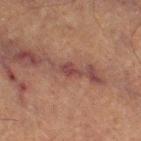follow-up: imaged on a skin check; not biopsied | automated metrics: a lesion area of about 3 mm² and an eccentricity of roughly 0.85; a detector confidence of about 85 out of 100 that the crop contains a lesion | acquisition: ~15 mm crop, total-body skin-cancer survey | subject: female, approximately 60 years of age | body site: the right thigh | tile lighting: cross-polarized illumination.